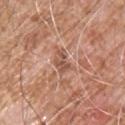Assessment:
Recorded during total-body skin imaging; not selected for excision or biopsy.
Background:
Approximately 3 mm at its widest. Captured under white-light illumination. The lesion-visualizer software estimated an average lesion color of about L≈53 a*≈22 b*≈29 (CIELAB), about 10 CIELAB-L* units darker than the surrounding skin, and a normalized lesion–skin contrast near 6.5. The analysis additionally found a border-irregularity index near 4.5/10, a within-lesion color-variation index near 3/10, and a peripheral color-asymmetry measure near 1. From the chest. A male subject roughly 55 years of age. A 15 mm close-up extracted from a 3D total-body photography capture.